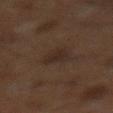Imaged during a routine full-body skin examination; the lesion was not biopsied and no histopathology is available. Measured at roughly 3 mm in maximum diameter. Automated tile analysis of the lesion measured a mean CIELAB color near L≈23 a*≈12 b*≈19, roughly 5 lightness units darker than nearby skin, and a normalized lesion–skin contrast near 6. It also reported a color-variation rating of about 2/10 and peripheral color asymmetry of about 1. The analysis additionally found lesion-presence confidence of about 100/100. A male patient, aged 58 to 62. Located on the mid back. This is a cross-polarized tile. A 15 mm close-up extracted from a 3D total-body photography capture.